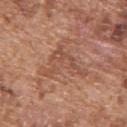workup=no biopsy performed (imaged during a skin exam); imaging modality=~15 mm crop, total-body skin-cancer survey; tile lighting=white-light; site=the upper back; subject=male, in their mid-70s.